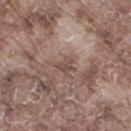Case summary:
– lighting · white-light
– automated metrics · a lesion area of about 3.5 mm², an eccentricity of roughly 0.6, and a shape-asymmetry score of about 0.45 (0 = symmetric); an average lesion color of about L≈48 a*≈17 b*≈23 (CIELAB), a lesion–skin lightness drop of about 8, and a normalized lesion–skin contrast near 6.5; a border-irregularity index near 4.5/10 and a peripheral color-asymmetry measure near 0.5
– lesion size · ≈3 mm
– acquisition · ~15 mm tile from a whole-body skin photo
– anatomic site · the right thigh
– subject · male, roughly 75 years of age